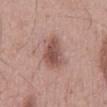Q: Is there a histopathology result?
A: imaged on a skin check; not biopsied
Q: What did automated image analysis measure?
A: about 12 CIELAB-L* units darker than the surrounding skin and a normalized border contrast of about 8.5; an automated nevus-likeness rating near 60 out of 100 and a lesion-detection confidence of about 100/100
Q: What kind of image is this?
A: 15 mm crop, total-body photography
Q: How large is the lesion?
A: about 5 mm
Q: What is the anatomic site?
A: the mid back
Q: What are the patient's age and sex?
A: male, in their mid- to late 50s